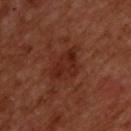notes: total-body-photography surveillance lesion; no biopsy | imaging modality: ~15 mm crop, total-body skin-cancer survey | image-analysis metrics: a lesion color around L≈26 a*≈25 b*≈27 in CIELAB and a normalized lesion–skin contrast near 7; a border-irregularity index near 3/10 | illumination: cross-polarized | subject: male, aged approximately 50 | lesion size: ~4.5 mm (longest diameter) | site: the upper back.